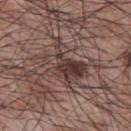{"biopsy_status": "not biopsied; imaged during a skin examination", "patient": {"sex": "male", "age_approx": 70}, "site": "back", "image": {"source": "total-body photography crop", "field_of_view_mm": 15}, "automated_metrics": {"border_irregularity_0_10": 7.0, "color_variation_0_10": 7.5, "peripheral_color_asymmetry": 3.0}, "lesion_size": {"long_diameter_mm_approx": 5.5}, "lighting": "white-light"}